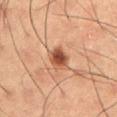Acquisition and patient details:
A male patient, aged 58 to 62. Measured at roughly 3.5 mm in maximum diameter. The tile uses cross-polarized illumination. This image is a 15 mm lesion crop taken from a total-body photograph. Automated image analysis of the tile measured a footprint of about 6 mm², an eccentricity of roughly 0.7, and a shape-asymmetry score of about 0.2 (0 = symmetric). And it measured a nevus-likeness score of about 95/100 and lesion-presence confidence of about 100/100.